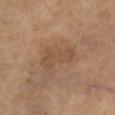  biopsy_status: not biopsied; imaged during a skin examination
  site: left lower leg
  lesion_size:
    long_diameter_mm_approx: 4.5
  image:
    source: total-body photography crop
    field_of_view_mm: 15
  automated_metrics:
    area_mm2_approx: 8.0
    eccentricity: 0.9
    shape_asymmetry: 0.35
    cielab_L: 46
    cielab_a: 17
    cielab_b: 30
    vs_skin_darker_L: 6.0
    vs_skin_contrast_norm: 5.0
    border_irregularity_0_10: 4.5
    color_variation_0_10: 2.0
    peripheral_color_asymmetry: 0.5
  patient:
    sex: female
    age_approx: 70
  lighting: cross-polarized